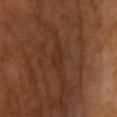<lesion>
  <biopsy_status>not biopsied; imaged during a skin examination</biopsy_status>
  <automated_metrics>
    <area_mm2_approx>3.5</area_mm2_approx>
    <eccentricity>0.85</eccentricity>
    <shape_asymmetry>0.35</shape_asymmetry>
    <nevus_likeness_0_100>0</nevus_likeness_0_100>
    <lesion_detection_confidence_0_100>80</lesion_detection_confidence_0_100>
  </automated_metrics>
  <site>right upper arm</site>
  <lighting>cross-polarized</lighting>
  <patient>
    <sex>female</sex>
    <age_approx>55</age_approx>
  </patient>
  <lesion_size>
    <long_diameter_mm_approx>3.0</long_diameter_mm_approx>
  </lesion_size>
  <image>
    <source>total-body photography crop</source>
    <field_of_view_mm>15</field_of_view_mm>
  </image>
</lesion>